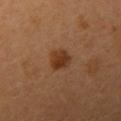Findings:
* workup: total-body-photography surveillance lesion; no biopsy
* automated lesion analysis: an area of roughly 5.5 mm²; a border-irregularity index near 1.5/10, a within-lesion color-variation index near 3.5/10, and radial color variation of about 1
* lighting: cross-polarized illumination
* size: ≈2.5 mm
* patient: female, roughly 35 years of age
* body site: the left upper arm
* image source: ~15 mm crop, total-body skin-cancer survey